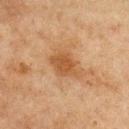Impression:
This lesion was catalogued during total-body skin photography and was not selected for biopsy.
Clinical summary:
About 4 mm across. A male subject, about 75 years old. A region of skin cropped from a whole-body photographic capture, roughly 15 mm wide. Located on the front of the torso.